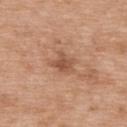image-analysis metrics — an automated nevus-likeness rating near 35 out of 100 and lesion-presence confidence of about 100/100
tile lighting — white-light
subject — male, in their 50s
acquisition — total-body-photography crop, ~15 mm field of view
lesion diameter — ~2.5 mm (longest diameter)
body site — the upper back